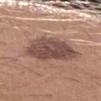Case summary:
* notes · total-body-photography surveillance lesion; no biopsy
* patient · male, aged approximately 25
* size · about 5.5 mm
* acquisition · 15 mm crop, total-body photography
* location · the left upper arm